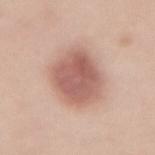Q: Was a biopsy performed?
A: catalogued during a skin exam; not biopsied